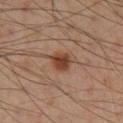The tile uses cross-polarized illumination.
A male subject aged 53 to 57.
Longest diameter approximately 2.5 mm.
From the right lower leg.
An algorithmic analysis of the crop reported a lesion area of about 5 mm², an eccentricity of roughly 0.65, and two-axis asymmetry of about 0.25. The analysis additionally found an automated nevus-likeness rating near 95 out of 100 and a lesion-detection confidence of about 100/100.
Cropped from a whole-body photographic skin survey; the tile spans about 15 mm.